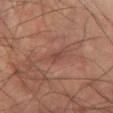workup = no biopsy performed (imaged during a skin exam).